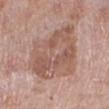workup: total-body-photography surveillance lesion; no biopsy
image: total-body-photography crop, ~15 mm field of view
subject: female, approximately 55 years of age
anatomic site: the left lower leg